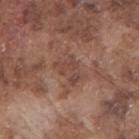Part of a total-body skin-imaging series; this lesion was reviewed on a skin check and was not flagged for biopsy. A male subject in their mid- to late 70s. A region of skin cropped from a whole-body photographic capture, roughly 15 mm wide. The lesion-visualizer software estimated a footprint of about 6 mm² and an eccentricity of roughly 0.7. The software also gave a lesion color around L≈45 a*≈20 b*≈25 in CIELAB, roughly 7 lightness units darker than nearby skin, and a normalized lesion–skin contrast near 5.5. The analysis additionally found a border-irregularity rating of about 6.5/10 and a color-variation rating of about 2/10. And it measured a classifier nevus-likeness of about 0/100 and lesion-presence confidence of about 80/100. Located on the right upper arm. The tile uses white-light illumination.